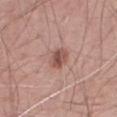Impression:
Recorded during total-body skin imaging; not selected for excision or biopsy.
Acquisition and patient details:
From the abdomen. The total-body-photography lesion software estimated a lesion area of about 5.5 mm², an outline eccentricity of about 0.7 (0 = round, 1 = elongated), and a symmetry-axis asymmetry near 0.25. And it measured roughly 11 lightness units darker than nearby skin and a normalized lesion–skin contrast near 7.5. The software also gave a border-irregularity rating of about 2/10, a within-lesion color-variation index near 4.5/10, and radial color variation of about 1.5. This is a white-light tile. Approximately 3 mm at its widest. A male patient, aged 48 to 52. A 15 mm close-up tile from a total-body photography series done for melanoma screening.